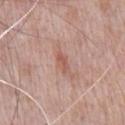| key | value |
|---|---|
| notes | total-body-photography surveillance lesion; no biopsy |
| patient | male, aged approximately 70 |
| body site | the chest |
| image | 15 mm crop, total-body photography |